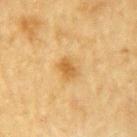| key | value |
|---|---|
| biopsy status | no biopsy performed (imaged during a skin exam) |
| lighting | cross-polarized illumination |
| subject | male, in their mid-80s or older |
| size | about 2.5 mm |
| imaging modality | total-body-photography crop, ~15 mm field of view |
| anatomic site | the left upper arm |